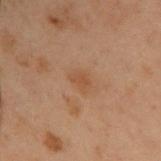Impression: The lesion was photographed on a routine skin check and not biopsied; there is no pathology result. Acquisition and patient details: A male patient, aged around 55. Cropped from a whole-body photographic skin survey; the tile spans about 15 mm. From the upper back. The total-body-photography lesion software estimated an average lesion color of about L≈40 a*≈18 b*≈28 (CIELAB), a lesion–skin lightness drop of about 5, and a lesion-to-skin contrast of about 5 (normalized; higher = more distinct). The analysis additionally found border irregularity of about 3.5 on a 0–10 scale, a color-variation rating of about 1/10, and peripheral color asymmetry of about 0.5. The analysis additionally found a classifier nevus-likeness of about 10/100.